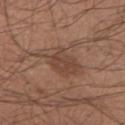Clinical impression:
Recorded during total-body skin imaging; not selected for excision or biopsy.
Context:
The lesion's longest dimension is about 5 mm. The patient is a male aged 28–32. Located on the arm. Cropped from a total-body skin-imaging series; the visible field is about 15 mm.